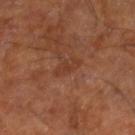biopsy status=imaged on a skin check; not biopsied
body site=the leg
illumination=cross-polarized
acquisition=~15 mm crop, total-body skin-cancer survey
diameter=about 3.5 mm
patient=male, roughly 60 years of age